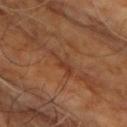| field | value |
|---|---|
| follow-up | imaged on a skin check; not biopsied |
| size | ~3.5 mm (longest diameter) |
| image | ~15 mm tile from a whole-body skin photo |
| illumination | cross-polarized |
| patient | male, aged approximately 60 |
| location | the chest |